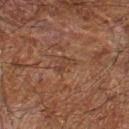{
  "biopsy_status": "not biopsied; imaged during a skin examination",
  "lesion_size": {
    "long_diameter_mm_approx": 2.5
  },
  "image": {
    "source": "total-body photography crop",
    "field_of_view_mm": 15
  },
  "site": "arm",
  "automated_metrics": {
    "area_mm2_approx": 3.5,
    "shape_asymmetry": 0.5,
    "border_irregularity_0_10": 6.0,
    "color_variation_0_10": 0.0,
    "peripheral_color_asymmetry": 0.0
  },
  "patient": {
    "sex": "male",
    "age_approx": 65
  },
  "lighting": "cross-polarized"
}